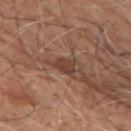Clinical impression: The lesion was photographed on a routine skin check and not biopsied; there is no pathology result. Image and clinical context: The lesion is located on the upper back. A lesion tile, about 15 mm wide, cut from a 3D total-body photograph. The patient is a male in their 60s. Captured under white-light illumination. The recorded lesion diameter is about 3.5 mm.